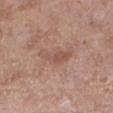workup: catalogued during a skin exam; not biopsied | illumination: white-light illumination | lesion size: about 4.5 mm | site: the left lower leg | image: ~15 mm tile from a whole-body skin photo | subject: female, about 50 years old.